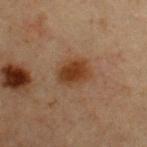Clinical impression:
Captured during whole-body skin photography for melanoma surveillance; the lesion was not biopsied.
Background:
The patient is a male about 60 years old. From the front of the torso. This is a cross-polarized tile. A 15 mm close-up tile from a total-body photography series done for melanoma screening.